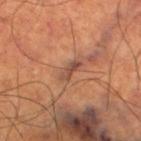Assessment: The lesion was photographed on a routine skin check and not biopsied; there is no pathology result. Context: Cropped from a total-body skin-imaging series; the visible field is about 15 mm. The lesion is on the right thigh. A male patient, in their 70s. Imaged with cross-polarized lighting.